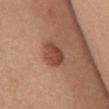The lesion was tiled from a total-body skin photograph and was not biopsied. Approximately 3.5 mm at its widest. From the chest. A female subject, aged approximately 55. A lesion tile, about 15 mm wide, cut from a 3D total-body photograph.